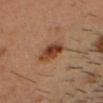Clinical impression: Part of a total-body skin-imaging series; this lesion was reviewed on a skin check and was not flagged for biopsy. Acquisition and patient details: The subject is a male aged around 35. A 15 mm crop from a total-body photograph taken for skin-cancer surveillance. Located on the head or neck.